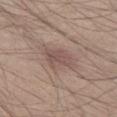follow-up: catalogued during a skin exam; not biopsied
body site: the left thigh
tile lighting: white-light illumination
subject: male, in their mid-20s
image: ~15 mm crop, total-body skin-cancer survey